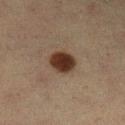{
  "biopsy_status": "not biopsied; imaged during a skin examination",
  "image": {
    "source": "total-body photography crop",
    "field_of_view_mm": 15
  },
  "automated_metrics": {
    "nevus_likeness_0_100": 100
  },
  "lighting": "cross-polarized",
  "lesion_size": {
    "long_diameter_mm_approx": 3.0
  },
  "patient": {
    "sex": "female",
    "age_approx": 55
  },
  "site": "left lower leg"
}